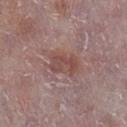Clinical impression:
Part of a total-body skin-imaging series; this lesion was reviewed on a skin check and was not flagged for biopsy.
Clinical summary:
This is a white-light tile. Measured at roughly 3.5 mm in maximum diameter. A 15 mm crop from a total-body photograph taken for skin-cancer surveillance. A male subject about 75 years old. Located on the left lower leg.